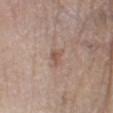Findings:
- workup — total-body-photography surveillance lesion; no biopsy
- image — total-body-photography crop, ~15 mm field of view
- automated metrics — a border-irregularity index near 4/10 and a within-lesion color-variation index near 1.5/10
- site — the right lower leg
- patient — female, aged approximately 75
- lighting — white-light illumination